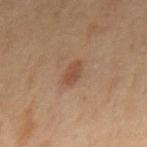biopsy status: no biopsy performed (imaged during a skin exam)
lesion size: ≈3 mm
site: the mid back
acquisition: ~15 mm crop, total-body skin-cancer survey
patient: male, aged 68 to 72
illumination: cross-polarized